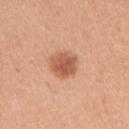Imaged during a routine full-body skin examination; the lesion was not biopsied and no histopathology is available. On the right upper arm. Cropped from a total-body skin-imaging series; the visible field is about 15 mm. A female patient, aged around 45. Imaged with white-light lighting. About 3.5 mm across.